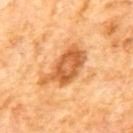Part of a total-body skin-imaging series; this lesion was reviewed on a skin check and was not flagged for biopsy.
Captured under cross-polarized illumination.
A lesion tile, about 15 mm wide, cut from a 3D total-body photograph.
Automated tile analysis of the lesion measured a shape-asymmetry score of about 0.35 (0 = symmetric).
The subject is a male approximately 65 years of age.
From the mid back.